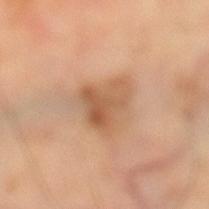{
  "biopsy_status": "not biopsied; imaged during a skin examination",
  "lighting": "cross-polarized",
  "patient": {
    "sex": "female",
    "age_approx": 60
  },
  "site": "leg",
  "lesion_size": {
    "long_diameter_mm_approx": 4.0
  },
  "automated_metrics": {
    "cielab_L": 58,
    "cielab_a": 22,
    "cielab_b": 35,
    "vs_skin_contrast_norm": 7.0,
    "border_irregularity_0_10": 4.5,
    "color_variation_0_10": 5.5,
    "peripheral_color_asymmetry": 2.0,
    "nevus_likeness_0_100": 5,
    "lesion_detection_confidence_0_100": 100
  },
  "image": {
    "source": "total-body photography crop",
    "field_of_view_mm": 15
  }
}